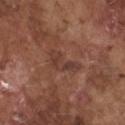The lesion was tiled from a total-body skin photograph and was not biopsied.
An algorithmic analysis of the crop reported a lesion area of about 6 mm², a shape eccentricity near 0.9, and two-axis asymmetry of about 0.55. The software also gave a mean CIELAB color near L≈39 a*≈21 b*≈25, roughly 7 lightness units darker than nearby skin, and a normalized border contrast of about 6. It also reported an automated nevus-likeness rating near 0 out of 100 and a lesion-detection confidence of about 95/100.
A male patient aged 73 to 77.
Located on the chest.
A lesion tile, about 15 mm wide, cut from a 3D total-body photograph.